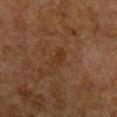Captured during whole-body skin photography for melanoma surveillance; the lesion was not biopsied.
Longest diameter approximately 3 mm.
A close-up tile cropped from a whole-body skin photograph, about 15 mm across.
From the chest.
A male subject in their 50s.
Captured under cross-polarized illumination.
The total-body-photography lesion software estimated a mean CIELAB color near L≈26 a*≈16 b*≈26 and roughly 4 lightness units darker than nearby skin. It also reported a border-irregularity index near 3.5/10, a color-variation rating of about 0.5/10, and peripheral color asymmetry of about 0. The analysis additionally found a lesion-detection confidence of about 100/100.